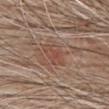Case summary:
• workup — catalogued during a skin exam; not biopsied
• body site — the chest
• subject — male, about 80 years old
• acquisition — total-body-photography crop, ~15 mm field of view